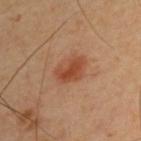Q: Is there a histopathology result?
A: no biopsy performed (imaged during a skin exam)
Q: Patient demographics?
A: male, aged 63–67
Q: What kind of image is this?
A: ~15 mm crop, total-body skin-cancer survey
Q: What lighting was used for the tile?
A: cross-polarized
Q: How large is the lesion?
A: ≈3.5 mm
Q: Lesion location?
A: the upper back